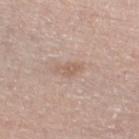This lesion was catalogued during total-body skin photography and was not selected for biopsy. A 15 mm crop from a total-body photograph taken for skin-cancer surveillance. A male patient aged 68–72. The lesion is located on the left lower leg.